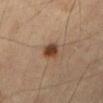lesion diameter: ≈3 mm; subject: male, aged 68 to 72; imaging modality: 15 mm crop, total-body photography; body site: the right thigh; tile lighting: cross-polarized illumination.